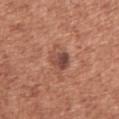Context: The lesion is on the right upper arm. This image is a 15 mm lesion crop taken from a total-body photograph. A female subject, roughly 50 years of age.